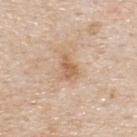Clinical impression:
No biopsy was performed on this lesion — it was imaged during a full skin examination and was not determined to be concerning.
Acquisition and patient details:
Imaged with white-light lighting. The lesion's longest dimension is about 3 mm. From the back. A region of skin cropped from a whole-body photographic capture, roughly 15 mm wide. A male subject aged approximately 45.